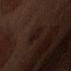Background: This is a white-light tile. A region of skin cropped from a whole-body photographic capture, roughly 15 mm wide. The total-body-photography lesion software estimated a mean CIELAB color near L≈15 a*≈15 b*≈17, about 4 CIELAB-L* units darker than the surrounding skin, and a lesion-to-skin contrast of about 6.5 (normalized; higher = more distinct). It also reported a border-irregularity index near 2.5/10, a color-variation rating of about 2.5/10, and peripheral color asymmetry of about 1. The software also gave an automated nevus-likeness rating near 0 out of 100 and lesion-presence confidence of about 100/100. A male subject roughly 70 years of age. The lesion is located on the abdomen.